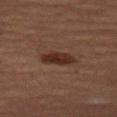The lesion was photographed on a routine skin check and not biopsied; there is no pathology result.
Captured under cross-polarized illumination.
A region of skin cropped from a whole-body photographic capture, roughly 15 mm wide.
From the left thigh.
Approximately 4.5 mm at its widest.
Automated image analysis of the tile measured an area of roughly 7.5 mm² and a shape eccentricity near 0.9. It also reported a lesion color around L≈28 a*≈17 b*≈23 in CIELAB, about 9 CIELAB-L* units darker than the surrounding skin, and a normalized lesion–skin contrast near 9.5. And it measured a border-irregularity rating of about 2/10, internal color variation of about 3 on a 0–10 scale, and a peripheral color-asymmetry measure near 1.
A female patient, aged 58–62.